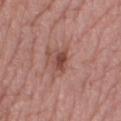biopsy status=no biopsy performed (imaged during a skin exam)
patient=female, roughly 70 years of age
image=~15 mm crop, total-body skin-cancer survey
site=the right thigh
TBP lesion metrics=a border-irregularity rating of about 3/10 and peripheral color asymmetry of about 0.5; a classifier nevus-likeness of about 70/100 and a detector confidence of about 100 out of 100 that the crop contains a lesion